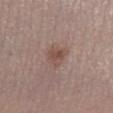No biopsy was performed on this lesion — it was imaged during a full skin examination and was not determined to be concerning. On the left lower leg. Imaged with white-light lighting. A 15 mm close-up extracted from a 3D total-body photography capture. A male subject, aged 48–52.